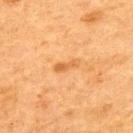This lesion was catalogued during total-body skin photography and was not selected for biopsy.
The patient is a male aged approximately 75.
Imaged with cross-polarized lighting.
A 15 mm close-up tile from a total-body photography series done for melanoma screening.
From the back.